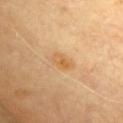Recorded during total-body skin imaging; not selected for excision or biopsy.
The lesion is located on the chest.
A close-up tile cropped from a whole-body skin photograph, about 15 mm across.
The lesion-visualizer software estimated a footprint of about 3.5 mm², an eccentricity of roughly 0.8, and two-axis asymmetry of about 0.2. The analysis additionally found roughly 8 lightness units darker than nearby skin and a normalized lesion–skin contrast near 6.5. The analysis additionally found a classifier nevus-likeness of about 25/100 and a lesion-detection confidence of about 100/100.
The subject is a male aged 63 to 67.